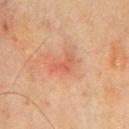Clinical impression:
Part of a total-body skin-imaging series; this lesion was reviewed on a skin check and was not flagged for biopsy.
Background:
The patient is a male aged 68 to 72. A lesion tile, about 15 mm wide, cut from a 3D total-body photograph. The lesion is on the front of the torso.